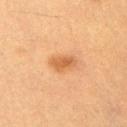Clinical impression: The lesion was photographed on a routine skin check and not biopsied; there is no pathology result. Image and clinical context: A male subject, aged 53–57. The lesion is on the arm. This image is a 15 mm lesion crop taken from a total-body photograph. Automated tile analysis of the lesion measured a shape eccentricity near 0.8 and a shape-asymmetry score of about 0.2 (0 = symmetric). The software also gave a border-irregularity index near 2/10 and radial color variation of about 1. The recorded lesion diameter is about 3.5 mm. This is a cross-polarized tile.